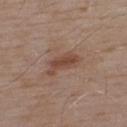Case summary:
• workup — imaged on a skin check; not biopsied
• image — ~15 mm crop, total-body skin-cancer survey
• subject — male, approximately 55 years of age
• body site — the upper back
• size — ≈4.5 mm